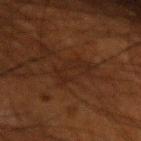Imaged during a routine full-body skin examination; the lesion was not biopsied and no histopathology is available.
Automated tile analysis of the lesion measured an average lesion color of about L≈18 a*≈15 b*≈21 (CIELAB), about 3 CIELAB-L* units darker than the surrounding skin, and a lesion-to-skin contrast of about 5 (normalized; higher = more distinct). The software also gave lesion-presence confidence of about 80/100.
Located on the left upper arm.
The patient is a male aged 48–52.
Measured at roughly 4 mm in maximum diameter.
This image is a 15 mm lesion crop taken from a total-body photograph.